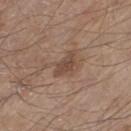notes — imaged on a skin check; not biopsied | body site — the left thigh | imaging modality — 15 mm crop, total-body photography | lesion diameter — ≈3 mm | automated lesion analysis — a footprint of about 4 mm², an outline eccentricity of about 0.85 (0 = round, 1 = elongated), and a shape-asymmetry score of about 0.3 (0 = symmetric); border irregularity of about 3 on a 0–10 scale, a within-lesion color-variation index near 2/10, and radial color variation of about 0.5 | tile lighting — white-light | subject — male, aged around 80.